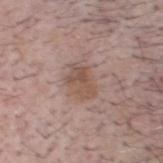| key | value |
|---|---|
| image-analysis metrics | a symmetry-axis asymmetry near 0.25; a border-irregularity rating of about 2.5/10, internal color variation of about 4.5 on a 0–10 scale, and radial color variation of about 1.5; a nevus-likeness score of about 20/100 and a detector confidence of about 100 out of 100 that the crop contains a lesion |
| subject | male, about 60 years old |
| site | the head or neck |
| image source | ~15 mm crop, total-body skin-cancer survey |